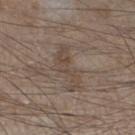Clinical impression: Captured during whole-body skin photography for melanoma surveillance; the lesion was not biopsied. Clinical summary: A 15 mm crop from a total-body photograph taken for skin-cancer surveillance. Automated tile analysis of the lesion measured an area of roughly 8 mm², a shape eccentricity near 0.95, and two-axis asymmetry of about 0.55. The software also gave a border-irregularity rating of about 7.5/10, internal color variation of about 2.5 on a 0–10 scale, and a peripheral color-asymmetry measure near 1. On the left lower leg. The subject is a male aged 43 to 47. This is a white-light tile.